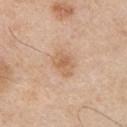* workup · imaged on a skin check; not biopsied
* lesion diameter · about 3 mm
* anatomic site · the chest
* patient · male, aged approximately 55
* lighting · white-light
* automated metrics · a border-irregularity rating of about 3/10, internal color variation of about 2.5 on a 0–10 scale, and peripheral color asymmetry of about 0.5
* imaging modality · ~15 mm crop, total-body skin-cancer survey